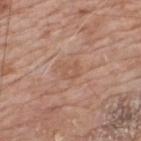Image and clinical context: Measured at roughly 3 mm in maximum diameter. A male patient, approximately 60 years of age. Located on the back. A roughly 15 mm field-of-view crop from a total-body skin photograph.